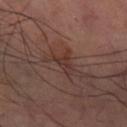Clinical impression: The lesion was tiled from a total-body skin photograph and was not biopsied. Image and clinical context: From the right thigh. This is a cross-polarized tile. A close-up tile cropped from a whole-body skin photograph, about 15 mm across.